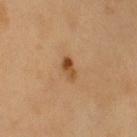{
  "patient": {
    "sex": "male",
    "age_approx": 60
  },
  "image": {
    "source": "total-body photography crop",
    "field_of_view_mm": 15
  },
  "site": "left upper arm"
}